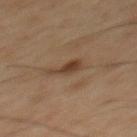| key | value |
|---|---|
| notes | total-body-photography surveillance lesion; no biopsy |
| patient | male, in their mid-40s |
| image source | total-body-photography crop, ~15 mm field of view |
| image-analysis metrics | a footprint of about 4 mm², an eccentricity of roughly 0.85, and two-axis asymmetry of about 0.45; a classifier nevus-likeness of about 90/100 and a lesion-detection confidence of about 100/100 |
| anatomic site | the upper back |